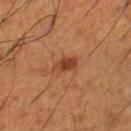Acquisition and patient details:
A lesion tile, about 15 mm wide, cut from a 3D total-body photograph. From the right thigh. The patient is a male roughly 65 years of age.